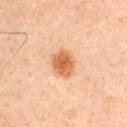{
  "biopsy_status": "not biopsied; imaged during a skin examination",
  "site": "left upper arm",
  "lesion_size": {
    "long_diameter_mm_approx": 3.5
  },
  "image": {
    "source": "total-body photography crop",
    "field_of_view_mm": 15
  },
  "lighting": "cross-polarized",
  "patient": {
    "sex": "male",
    "age_approx": 45
  }
}